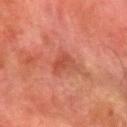Imaged during a routine full-body skin examination; the lesion was not biopsied and no histopathology is available. The patient is a male in their 80s. Captured under cross-polarized illumination. Automated image analysis of the tile measured a lesion area of about 5 mm² and two-axis asymmetry of about 0.4. And it measured an average lesion color of about L≈39 a*≈25 b*≈27 (CIELAB), a lesion–skin lightness drop of about 6, and a normalized border contrast of about 5.5. It also reported border irregularity of about 4.5 on a 0–10 scale, a within-lesion color-variation index near 4/10, and radial color variation of about 1.5. The analysis additionally found a lesion-detection confidence of about 100/100. The lesion is located on the arm. A 15 mm close-up tile from a total-body photography series done for melanoma screening.